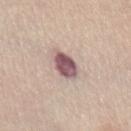Findings:
- workup: total-body-photography surveillance lesion; no biopsy
- body site: the abdomen
- automated metrics: an average lesion color of about L≈55 a*≈20 b*≈17 (CIELAB), about 18 CIELAB-L* units darker than the surrounding skin, and a lesion-to-skin contrast of about 12 (normalized; higher = more distinct); an automated nevus-likeness rating near 0 out of 100
- image source: 15 mm crop, total-body photography
- lighting: white-light illumination
- patient: female, in their mid-50s